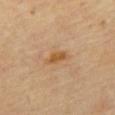<lesion>
<lesion_size>
  <long_diameter_mm_approx>3.0</long_diameter_mm_approx>
</lesion_size>
<site>chest</site>
<patient>
  <sex>female</sex>
  <age_approx>70</age_approx>
</patient>
<image>
  <source>total-body photography crop</source>
  <field_of_view_mm>15</field_of_view_mm>
</image>
</lesion>